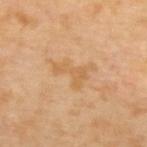Impression: Captured during whole-body skin photography for melanoma surveillance; the lesion was not biopsied. Background: The lesion's longest dimension is about 4.5 mm. A region of skin cropped from a whole-body photographic capture, roughly 15 mm wide. An algorithmic analysis of the crop reported a lesion–skin lightness drop of about 6 and a normalized lesion–skin contrast near 5.5. The software also gave border irregularity of about 8.5 on a 0–10 scale, a color-variation rating of about 0.5/10, and peripheral color asymmetry of about 0. On the upper back. The patient is a female roughly 65 years of age. Captured under cross-polarized illumination.